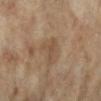notes=imaged on a skin check; not biopsied
automated metrics=border irregularity of about 6.5 on a 0–10 scale and radial color variation of about 0
patient=female, aged approximately 75
size=~3 mm (longest diameter)
acquisition=~15 mm crop, total-body skin-cancer survey
lighting=cross-polarized illumination
anatomic site=the right lower leg